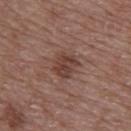Captured during whole-body skin photography for melanoma surveillance; the lesion was not biopsied. On the back. A female subject approximately 65 years of age. The tile uses white-light illumination. Cropped from a total-body skin-imaging series; the visible field is about 15 mm.